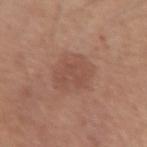Recorded during total-body skin imaging; not selected for excision or biopsy.
The patient is a male in their mid- to late 40s.
The lesion is located on the left upper arm.
This image is a 15 mm lesion crop taken from a total-body photograph.